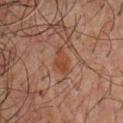notes: imaged on a skin check; not biopsied
image: total-body-photography crop, ~15 mm field of view
automated lesion analysis: a mean CIELAB color near L≈32 a*≈19 b*≈26 and about 6 CIELAB-L* units darker than the surrounding skin; a border-irregularity index near 3.5/10, internal color variation of about 1.5 on a 0–10 scale, and peripheral color asymmetry of about 0.5; an automated nevus-likeness rating near 70 out of 100 and a lesion-detection confidence of about 100/100
lesion diameter: ≈3 mm
subject: male, aged 58 to 62
tile lighting: cross-polarized
site: the chest